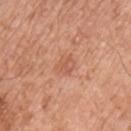Assessment: No biopsy was performed on this lesion — it was imaged during a full skin examination and was not determined to be concerning. Acquisition and patient details: From the chest. A male patient, roughly 75 years of age. The lesion-visualizer software estimated an average lesion color of about L≈58 a*≈25 b*≈33 (CIELAB), roughly 7 lightness units darker than nearby skin, and a lesion-to-skin contrast of about 5 (normalized; higher = more distinct). The analysis additionally found a border-irregularity rating of about 2/10, a color-variation rating of about 1.5/10, and radial color variation of about 0.5. The analysis additionally found a classifier nevus-likeness of about 0/100 and lesion-presence confidence of about 100/100. Captured under white-light illumination. Measured at roughly 2.5 mm in maximum diameter. A close-up tile cropped from a whole-body skin photograph, about 15 mm across.